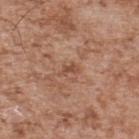biopsy status: total-body-photography surveillance lesion; no biopsy